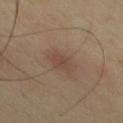The lesion was photographed on a routine skin check and not biopsied; there is no pathology result. On the chest. A 15 mm close-up extracted from a 3D total-body photography capture. A male subject, aged 38–42.